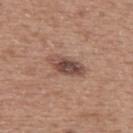notes = catalogued during a skin exam; not biopsied
subject = male, aged 58–62
location = the upper back
illumination = white-light illumination
acquisition = 15 mm crop, total-body photography
lesion diameter = ≈4.5 mm